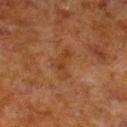Captured during whole-body skin photography for melanoma surveillance; the lesion was not biopsied. Measured at roughly 5 mm in maximum diameter. A 15 mm close-up extracted from a 3D total-body photography capture. From the right lower leg. This is a cross-polarized tile. A male patient roughly 80 years of age.